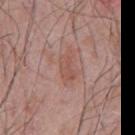Context:
A male patient about 60 years old. The tile uses white-light illumination. The lesion is on the abdomen. The recorded lesion diameter is about 3.5 mm. A 15 mm close-up tile from a total-body photography series done for melanoma screening. The lesion-visualizer software estimated a shape eccentricity near 0.85 and a symmetry-axis asymmetry near 0.35. The software also gave a border-irregularity rating of about 4/10, a color-variation rating of about 2/10, and a peripheral color-asymmetry measure near 0.5.